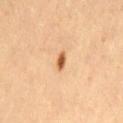Assessment:
This lesion was catalogued during total-body skin photography and was not selected for biopsy.
Background:
This is a cross-polarized tile. The lesion-visualizer software estimated a lesion area of about 2.5 mm² and a shape eccentricity near 0.8. And it measured a lesion color around L≈50 a*≈21 b*≈36 in CIELAB, about 14 CIELAB-L* units darker than the surrounding skin, and a normalized lesion–skin contrast near 10. The analysis additionally found a border-irregularity index near 2.5/10 and radial color variation of about 0.5. A 15 mm close-up extracted from a 3D total-body photography capture. Approximately 2 mm at its widest. A female patient roughly 40 years of age. On the lower back.